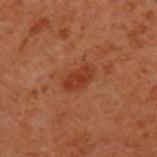Imaged during a routine full-body skin examination; the lesion was not biopsied and no histopathology is available. The tile uses cross-polarized illumination. The lesion-visualizer software estimated an area of roughly 5.5 mm², an outline eccentricity of about 0.85 (0 = round, 1 = elongated), and two-axis asymmetry of about 0.2. The analysis additionally found a lesion–skin lightness drop of about 6. The software also gave a border-irregularity index near 2/10 and a peripheral color-asymmetry measure near 0.5. It also reported an automated nevus-likeness rating near 75 out of 100. A male patient, aged 58 to 62. From the upper back. A 15 mm close-up tile from a total-body photography series done for melanoma screening.